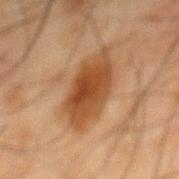| field | value |
|---|---|
| workup | total-body-photography surveillance lesion; no biopsy |
| site | the mid back |
| acquisition | ~15 mm crop, total-body skin-cancer survey |
| subject | male, aged 63 to 67 |
| automated lesion analysis | a lesion color around L≈38 a*≈20 b*≈32 in CIELAB, about 11 CIELAB-L* units darker than the surrounding skin, and a lesion-to-skin contrast of about 9.5 (normalized; higher = more distinct); a border-irregularity rating of about 2.5/10, a within-lesion color-variation index near 4/10, and radial color variation of about 1 |